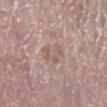Imaged during a routine full-body skin examination; the lesion was not biopsied and no histopathology is available. A region of skin cropped from a whole-body photographic capture, roughly 15 mm wide. The subject is a female aged 58–62. Located on the left lower leg.